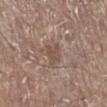Impression: Recorded during total-body skin imaging; not selected for excision or biopsy. Background: The lesion is located on the right lower leg. This is a white-light tile. A close-up tile cropped from a whole-body skin photograph, about 15 mm across. Measured at roughly 3 mm in maximum diameter. A male subject, aged approximately 70.